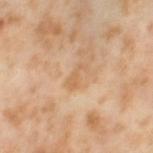Assessment: Imaged during a routine full-body skin examination; the lesion was not biopsied and no histopathology is available.